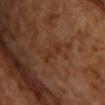{
  "biopsy_status": "not biopsied; imaged during a skin examination",
  "lighting": "cross-polarized",
  "lesion_size": {
    "long_diameter_mm_approx": 3.0
  },
  "image": {
    "source": "total-body photography crop",
    "field_of_view_mm": 15
  },
  "patient": {
    "sex": "female"
  },
  "automated_metrics": {
    "cielab_L": 33,
    "cielab_a": 22,
    "cielab_b": 30,
    "vs_skin_darker_L": 5.0,
    "border_irregularity_0_10": 4.0,
    "color_variation_0_10": 1.5
  },
  "site": "chest"
}